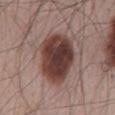Notes:
- notes — total-body-photography surveillance lesion; no biopsy
- lesion diameter — ≈6.5 mm
- tile lighting — white-light
- automated metrics — a footprint of about 24 mm²; internal color variation of about 5.5 on a 0–10 scale; a nevus-likeness score of about 60/100 and a detector confidence of about 100 out of 100 that the crop contains a lesion
- site — the mid back
- imaging modality — ~15 mm crop, total-body skin-cancer survey
- subject — male, aged around 65